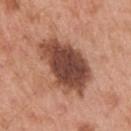biopsy status: no biopsy performed (imaged during a skin exam) | subject: male, aged approximately 55 | imaging modality: total-body-photography crop, ~15 mm field of view | lighting: white-light | anatomic site: the arm.